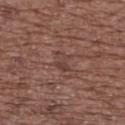lesion diameter: about 2.5 mm | patient: female, about 75 years old | TBP lesion metrics: a footprint of about 3.5 mm², an outline eccentricity of about 0.75 (0 = round, 1 = elongated), and a shape-asymmetry score of about 0.3 (0 = symmetric); a lesion color around L≈41 a*≈19 b*≈23 in CIELAB and about 7 CIELAB-L* units darker than the surrounding skin; a classifier nevus-likeness of about 0/100 and a lesion-detection confidence of about 90/100 | tile lighting: white-light | anatomic site: the upper back | image: 15 mm crop, total-body photography.